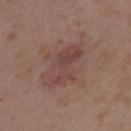The lesion was tiled from a total-body skin photograph and was not biopsied. A close-up tile cropped from a whole-body skin photograph, about 15 mm across. A female patient aged around 35. Longest diameter approximately 5.5 mm. Captured under white-light illumination. From the left upper arm.